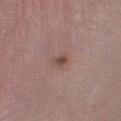No biopsy was performed on this lesion — it was imaged during a full skin examination and was not determined to be concerning. Cropped from a whole-body photographic skin survey; the tile spans about 15 mm. The lesion's longest dimension is about 1.5 mm. The patient is a male aged around 25. Located on the left lower leg. Imaged with white-light lighting.